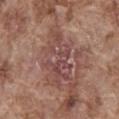| feature | finding |
|---|---|
| patient | male, aged approximately 75 |
| acquisition | total-body-photography crop, ~15 mm field of view |
| anatomic site | the abdomen |